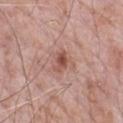<tbp_lesion>
<biopsy_status>not biopsied; imaged during a skin examination</biopsy_status>
<patient>
  <sex>male</sex>
  <age_approx>70</age_approx>
</patient>
<image>
  <source>total-body photography crop</source>
  <field_of_view_mm>15</field_of_view_mm>
</image>
<lesion_size>
  <long_diameter_mm_approx>3.0</long_diameter_mm_approx>
</lesion_size>
<site>chest</site>
</tbp_lesion>